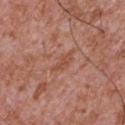{
  "biopsy_status": "not biopsied; imaged during a skin examination"
}